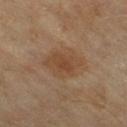Impression: Part of a total-body skin-imaging series; this lesion was reviewed on a skin check and was not flagged for biopsy. Clinical summary: The lesion is on the right thigh. A region of skin cropped from a whole-body photographic capture, roughly 15 mm wide. This is a cross-polarized tile. The subject is a female aged around 60.